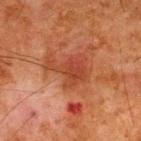Notes:
– notes · no biopsy performed (imaged during a skin exam)
– site · the upper back
– lesion diameter · ≈4.5 mm
– imaging modality · 15 mm crop, total-body photography
– subject · male, about 65 years old